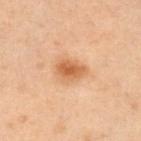follow-up: imaged on a skin check; not biopsied | TBP lesion metrics: an eccentricity of roughly 0.65 and a symmetry-axis asymmetry near 0.3; a nevus-likeness score of about 90/100 and lesion-presence confidence of about 100/100 | tile lighting: cross-polarized illumination | subject: male, approximately 50 years of age | body site: the right upper arm | imaging modality: ~15 mm tile from a whole-body skin photo | diameter: ~3.5 mm (longest diameter).